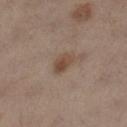follow-up = total-body-photography surveillance lesion; no biopsy | imaging modality = ~15 mm crop, total-body skin-cancer survey | lesion size = ≈2.5 mm | location = the left leg | lighting = cross-polarized | subject = female, aged 53 to 57.